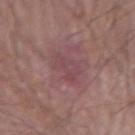biopsy status: no biopsy performed (imaged during a skin exam) | patient: male, approximately 65 years of age | automated lesion analysis: a shape eccentricity near 0.6 and a symmetry-axis asymmetry near 0.3; an average lesion color of about L≈45 a*≈23 b*≈16 (CIELAB), about 6 CIELAB-L* units darker than the surrounding skin, and a lesion-to-skin contrast of about 5 (normalized; higher = more distinct); a nevus-likeness score of about 0/100 and a lesion-detection confidence of about 85/100 | site: the left forearm | diameter: ≈3.5 mm | illumination: white-light | acquisition: 15 mm crop, total-body photography.